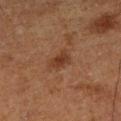follow-up: no biopsy performed (imaged during a skin exam); subject: male, aged 73–77; image source: total-body-photography crop, ~15 mm field of view; site: the leg; lighting: cross-polarized.